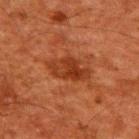<case>
<biopsy_status>not biopsied; imaged during a skin examination</biopsy_status>
<site>upper back</site>
<image>
  <source>total-body photography crop</source>
  <field_of_view_mm>15</field_of_view_mm>
</image>
<patient>
  <sex>male</sex>
  <age_approx>60</age_approx>
</patient>
</case>